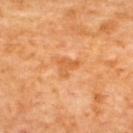<case>
  <biopsy_status>not biopsied; imaged during a skin examination</biopsy_status>
  <patient>
    <sex>female</sex>
    <age_approx>65</age_approx>
  </patient>
  <lighting>cross-polarized</lighting>
  <lesion_size>
    <long_diameter_mm_approx>3.0</long_diameter_mm_approx>
  </lesion_size>
  <image>
    <source>total-body photography crop</source>
    <field_of_view_mm>15</field_of_view_mm>
  </image>
  <automated_metrics>
    <area_mm2_approx>4.5</area_mm2_approx>
    <eccentricity>0.5</eccentricity>
    <shape_asymmetry>0.55</shape_asymmetry>
    <nevus_likeness_0_100>0</nevus_likeness_0_100>
    <lesion_detection_confidence_0_100>100</lesion_detection_confidence_0_100>
  </automated_metrics>
  <site>upper back</site>
</case>